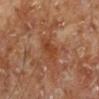{"patient": {"sex": "male", "age_approx": 70}, "site": "left lower leg", "image": {"source": "total-body photography crop", "field_of_view_mm": 15}}